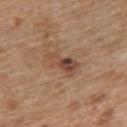Q: Was a biopsy performed?
A: imaged on a skin check; not biopsied
Q: What is the anatomic site?
A: the left upper arm
Q: Automated lesion metrics?
A: a lesion color around L≈47 a*≈19 b*≈29 in CIELAB, about 10 CIELAB-L* units darker than the surrounding skin, and a lesion-to-skin contrast of about 7.5 (normalized; higher = more distinct); border irregularity of about 4 on a 0–10 scale and internal color variation of about 9.5 on a 0–10 scale
Q: How was the tile lit?
A: white-light illumination
Q: How large is the lesion?
A: about 4 mm
Q: Patient demographics?
A: male, in their mid- to late 60s
Q: How was this image acquired?
A: total-body-photography crop, ~15 mm field of view